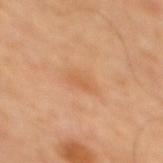<record>
  <image>
    <source>total-body photography crop</source>
    <field_of_view_mm>15</field_of_view_mm>
  </image>
  <automated_metrics>
    <area_mm2_approx>6.0</area_mm2_approx>
    <eccentricity>0.8</eccentricity>
    <shape_asymmetry>0.3</shape_asymmetry>
    <nevus_likeness_0_100>0</nevus_likeness_0_100>
    <lesion_detection_confidence_0_100>100</lesion_detection_confidence_0_100>
  </automated_metrics>
  <lighting>cross-polarized</lighting>
  <patient>
    <sex>male</sex>
    <age_approx>65</age_approx>
  </patient>
  <site>back</site>
</record>